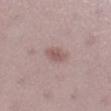Clinical impression: This lesion was catalogued during total-body skin photography and was not selected for biopsy. Background: Located on the right lower leg. The tile uses white-light illumination. Automated image analysis of the tile measured an average lesion color of about L≈54 a*≈19 b*≈20 (CIELAB). The analysis additionally found border irregularity of about 2.5 on a 0–10 scale, internal color variation of about 2 on a 0–10 scale, and peripheral color asymmetry of about 0.5. A female patient aged 23 to 27. Cropped from a whole-body photographic skin survey; the tile spans about 15 mm.